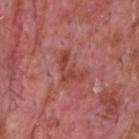biopsy status = no biopsy performed (imaged during a skin exam)
image = total-body-photography crop, ~15 mm field of view
lighting = white-light illumination
site = the head or neck
diameter = ~4.5 mm (longest diameter)
subject = male, aged around 60